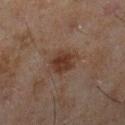Context:
The tile uses cross-polarized illumination. A close-up tile cropped from a whole-body skin photograph, about 15 mm across. A male subject in their mid- to late 40s. The recorded lesion diameter is about 3 mm. The lesion is located on the right lower leg.